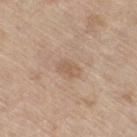Findings:
– follow-up · no biopsy performed (imaged during a skin exam)
– TBP lesion metrics · an outline eccentricity of about 0.8 (0 = round, 1 = elongated) and two-axis asymmetry of about 0.2; a mean CIELAB color near L≈58 a*≈16 b*≈30 and roughly 7 lightness units darker than nearby skin; a border-irregularity index near 2/10 and radial color variation of about 0.5
– lighting · white-light illumination
– subject · male, aged 68–72
– site · the leg
– acquisition · ~15 mm tile from a whole-body skin photo
– lesion size · ~3 mm (longest diameter)